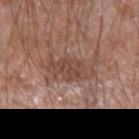{
  "biopsy_status": "not biopsied; imaged during a skin examination",
  "automated_metrics": {
    "cielab_L": 46,
    "cielab_a": 19,
    "cielab_b": 24,
    "vs_skin_darker_L": 8.0,
    "vs_skin_contrast_norm": 6.5,
    "nevus_likeness_0_100": 0,
    "lesion_detection_confidence_0_100": 100
  },
  "lighting": "white-light",
  "image": {
    "source": "total-body photography crop",
    "field_of_view_mm": 15
  },
  "patient": {
    "sex": "male",
    "age_approx": 60
  },
  "site": "left forearm"
}